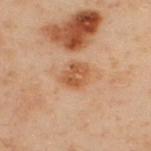workup: total-body-photography surveillance lesion; no biopsy
acquisition: ~15 mm tile from a whole-body skin photo
location: the upper back
illumination: cross-polarized
subject: male, aged around 50